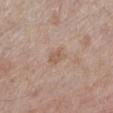Case summary:
– notes · total-body-photography surveillance lesion; no biopsy
– site · the leg
– size · ~2.5 mm (longest diameter)
– acquisition · total-body-photography crop, ~15 mm field of view
– tile lighting · white-light illumination
– subject · male, approximately 70 years of age
– TBP lesion metrics · a lesion area of about 3.5 mm², an eccentricity of roughly 0.7, and a shape-asymmetry score of about 0.35 (0 = symmetric); a border-irregularity rating of about 3/10 and radial color variation of about 0.5